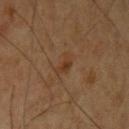Assessment: Captured during whole-body skin photography for melanoma surveillance; the lesion was not biopsied. Acquisition and patient details: Imaged with cross-polarized lighting. The lesion's longest dimension is about 2.5 mm. Located on the right upper arm. Automated tile analysis of the lesion measured a footprint of about 3 mm², an outline eccentricity of about 0.85 (0 = round, 1 = elongated), and two-axis asymmetry of about 0.5. And it measured a detector confidence of about 100 out of 100 that the crop contains a lesion. A male subject aged around 60. This image is a 15 mm lesion crop taken from a total-body photograph.